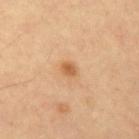No biopsy was performed on this lesion — it was imaged during a full skin examination and was not determined to be concerning. A lesion tile, about 15 mm wide, cut from a 3D total-body photograph. A male subject about 55 years old. From the left upper arm.